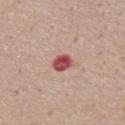{
  "biopsy_status": "not biopsied; imaged during a skin examination",
  "site": "chest",
  "patient": {
    "sex": "male",
    "age_approx": 55
  },
  "image": {
    "source": "total-body photography crop",
    "field_of_view_mm": 15
  }
}